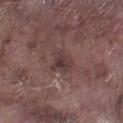This lesion was catalogued during total-body skin photography and was not selected for biopsy. About 3 mm across. The lesion-visualizer software estimated a lesion area of about 6.5 mm², a shape eccentricity near 0.35, and a symmetry-axis asymmetry near 0.35. The software also gave a border-irregularity index near 3.5/10, a within-lesion color-variation index near 4/10, and a peripheral color-asymmetry measure near 1. Imaged with white-light lighting. From the leg. A region of skin cropped from a whole-body photographic capture, roughly 15 mm wide. The subject is a male aged 73–77.